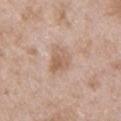Findings:
- notes · no biopsy performed (imaged during a skin exam)
- acquisition · 15 mm crop, total-body photography
- patient · male, in their 50s
- illumination · white-light illumination
- site · the chest
- automated metrics · a lesion area of about 7 mm² and a symmetry-axis asymmetry near 0.3; a border-irregularity rating of about 3.5/10, a within-lesion color-variation index near 4/10, and a peripheral color-asymmetry measure near 1.5; a classifier nevus-likeness of about 0/100 and a lesion-detection confidence of about 100/100
- size · ≈4 mm